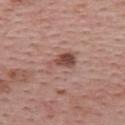Q: Was a biopsy performed?
A: catalogued during a skin exam; not biopsied
Q: How was this image acquired?
A: ~15 mm tile from a whole-body skin photo
Q: Automated lesion metrics?
A: an outline eccentricity of about 0.75 (0 = round, 1 = elongated) and a shape-asymmetry score of about 0.4 (0 = symmetric); a lesion-to-skin contrast of about 6.5 (normalized; higher = more distinct); border irregularity of about 5 on a 0–10 scale
Q: How large is the lesion?
A: about 4 mm
Q: Who is the patient?
A: female, in their mid-50s
Q: Illumination type?
A: white-light
Q: What is the anatomic site?
A: the back